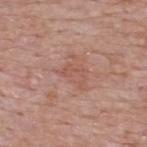Recorded during total-body skin imaging; not selected for excision or biopsy.
A 15 mm close-up tile from a total-body photography series done for melanoma screening.
This is a white-light tile.
A male subject, aged approximately 55.
The recorded lesion diameter is about 3 mm.
On the upper back.